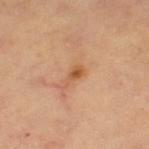A 15 mm close-up extracted from a 3D total-body photography capture. This is a cross-polarized tile. Longest diameter approximately 3 mm. The lesion is located on the left thigh. The subject is aged approximately 60. Automated image analysis of the tile measured a nevus-likeness score of about 10/100 and a detector confidence of about 100 out of 100 that the crop contains a lesion.